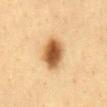biopsy status — imaged on a skin check; not biopsied | automated metrics — a border-irregularity rating of about 1.5/10, a within-lesion color-variation index near 6.5/10, and peripheral color asymmetry of about 2; an automated nevus-likeness rating near 100 out of 100 | anatomic site — the abdomen | subject — male, approximately 60 years of age | lesion size — ~4.5 mm (longest diameter) | lighting — cross-polarized | image — 15 mm crop, total-body photography.